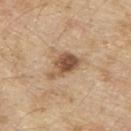Clinical impression:
No biopsy was performed on this lesion — it was imaged during a full skin examination and was not determined to be concerning.
Background:
A male patient roughly 70 years of age. Located on the upper back. A roughly 15 mm field-of-view crop from a total-body skin photograph.